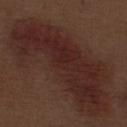• notes · total-body-photography surveillance lesion; no biopsy
• subject · male, roughly 70 years of age
• site · the leg
• lighting · white-light illumination
• lesion size · about 15 mm
• imaging modality · ~15 mm tile from a whole-body skin photo
• TBP lesion metrics · an automated nevus-likeness rating near 0 out of 100 and lesion-presence confidence of about 90/100